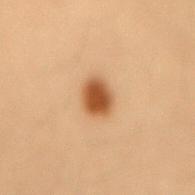body site: the back | lighting: cross-polarized | subject: female, aged 38–42 | automated metrics: a border-irregularity index near 1.5/10, internal color variation of about 3 on a 0–10 scale, and a peripheral color-asymmetry measure near 0.5; a nevus-likeness score of about 100/100 and a lesion-detection confidence of about 100/100 | image source: total-body-photography crop, ~15 mm field of view | lesion size: ≈3.5 mm.